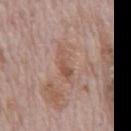biopsy_status: not biopsied; imaged during a skin examination